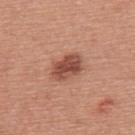Findings:
• lighting — white-light
• patient — male, aged 53–57
• acquisition — ~15 mm tile from a whole-body skin photo
• lesion size — about 4 mm
• location — the back